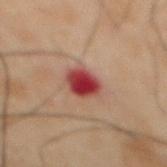Automated tile analysis of the lesion measured an outline eccentricity of about 0.55 (0 = round, 1 = elongated) and two-axis asymmetry of about 0.15. The software also gave a lesion–skin lightness drop of about 13 and a normalized border contrast of about 12. It also reported a border-irregularity rating of about 1.5/10, a within-lesion color-variation index near 4.5/10, and a peripheral color-asymmetry measure near 1.
Measured at roughly 3.5 mm in maximum diameter.
A region of skin cropped from a whole-body photographic capture, roughly 15 mm wide.
On the abdomen.
A male subject aged approximately 50.
The tile uses cross-polarized illumination.